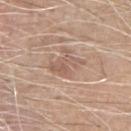This lesion was catalogued during total-body skin photography and was not selected for biopsy.
Cropped from a whole-body photographic skin survey; the tile spans about 15 mm.
The subject is a male about 65 years old.
Located on the right forearm.
Approximately 4 mm at its widest.